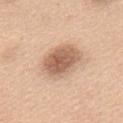No biopsy was performed on this lesion — it was imaged during a full skin examination and was not determined to be concerning. Captured under white-light illumination. Measured at roughly 5 mm in maximum diameter. From the upper back. The patient is a female aged around 55. A 15 mm close-up tile from a total-body photography series done for melanoma screening.